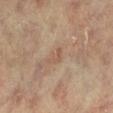Impression: This lesion was catalogued during total-body skin photography and was not selected for biopsy. Image and clinical context: A female patient, aged 78 to 82. On the left lower leg. Measured at roughly 2.5 mm in maximum diameter. A roughly 15 mm field-of-view crop from a total-body skin photograph. This is a cross-polarized tile.